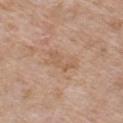Imaged during a routine full-body skin examination; the lesion was not biopsied and no histopathology is available. A 15 mm crop from a total-body photograph taken for skin-cancer surveillance. The tile uses white-light illumination. A male subject roughly 60 years of age. The lesion is on the chest. Measured at roughly 4 mm in maximum diameter. The lesion-visualizer software estimated a lesion area of about 5.5 mm², a shape eccentricity near 0.9, and two-axis asymmetry of about 0.5. It also reported roughly 6 lightness units darker than nearby skin and a normalized border contrast of about 4.5. The analysis additionally found radial color variation of about 0. The software also gave a classifier nevus-likeness of about 0/100 and a detector confidence of about 100 out of 100 that the crop contains a lesion.